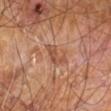| feature | finding |
|---|---|
| image-analysis metrics | an area of roughly 4 mm², a shape eccentricity near 0.9, and two-axis asymmetry of about 0.4; a border-irregularity index near 5/10 |
| illumination | cross-polarized |
| location | the right leg |
| patient | male, about 60 years old |
| image source | ~15 mm tile from a whole-body skin photo |
| size | ≈3.5 mm |